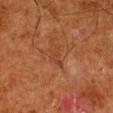Q: Was this lesion biopsied?
A: total-body-photography surveillance lesion; no biopsy
Q: Lesion location?
A: the leg
Q: How large is the lesion?
A: ~3 mm (longest diameter)
Q: Who is the patient?
A: male, roughly 80 years of age
Q: Illumination type?
A: cross-polarized
Q: What is the imaging modality?
A: ~15 mm tile from a whole-body skin photo